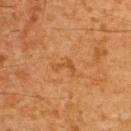Case summary:
• follow-up — catalogued during a skin exam; not biopsied
• acquisition — ~15 mm tile from a whole-body skin photo
• subject — male, roughly 60 years of age
• size — about 2.5 mm
• tile lighting — cross-polarized
• body site — the back